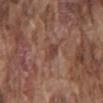Imaged during a routine full-body skin examination; the lesion was not biopsied and no histopathology is available. This is a white-light tile. The lesion is on the back. An algorithmic analysis of the crop reported a classifier nevus-likeness of about 0/100 and lesion-presence confidence of about 100/100. A male patient in their mid- to late 70s. About 2.5 mm across. A close-up tile cropped from a whole-body skin photograph, about 15 mm across.